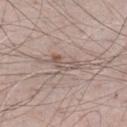Assessment: Imaged during a routine full-body skin examination; the lesion was not biopsied and no histopathology is available. Background: A male subject about 65 years old. Located on the abdomen. About 3.5 mm across. A roughly 15 mm field-of-view crop from a total-body skin photograph. The tile uses white-light illumination. The total-body-photography lesion software estimated a border-irregularity rating of about 6.5/10, a within-lesion color-variation index near 0/10, and peripheral color asymmetry of about 0.